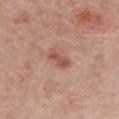The lesion was tiled from a total-body skin photograph and was not biopsied. A region of skin cropped from a whole-body photographic capture, roughly 15 mm wide. Longest diameter approximately 3.5 mm. Imaged with white-light lighting. The lesion is on the chest. The patient is a female in their 50s.